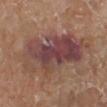Recorded during total-body skin imaging; not selected for excision or biopsy. About 10.5 mm across. A female patient in their mid- to late 70s. Cropped from a total-body skin-imaging series; the visible field is about 15 mm. Automated tile analysis of the lesion measured a mean CIELAB color near L≈43 a*≈20 b*≈21, about 11 CIELAB-L* units darker than the surrounding skin, and a lesion-to-skin contrast of about 10 (normalized; higher = more distinct). The software also gave a classifier nevus-likeness of about 5/100. This is a white-light tile. The lesion is on the left lower leg.